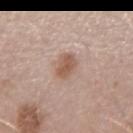biopsy status: no biopsy performed (imaged during a skin exam)
image: 15 mm crop, total-body photography
patient: female, roughly 45 years of age
illumination: white-light illumination
site: the left forearm